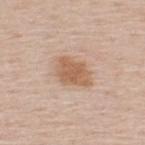notes = no biopsy performed (imaged during a skin exam)
subject = male, in their 60s
image source = total-body-photography crop, ~15 mm field of view
site = the upper back
lighting = white-light illumination
automated lesion analysis = a lesion area of about 10 mm² and an eccentricity of roughly 0.75; a border-irregularity rating of about 2.5/10 and a within-lesion color-variation index near 2.5/10; a nevus-likeness score of about 70/100 and a lesion-detection confidence of about 100/100